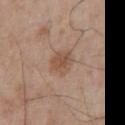notes: total-body-photography surveillance lesion; no biopsy
patient: male, roughly 65 years of age
site: the chest
lesion diameter: ≈3.5 mm
lighting: white-light
acquisition: ~15 mm tile from a whole-body skin photo
image-analysis metrics: a lesion area of about 7 mm², an eccentricity of roughly 0.65, and a shape-asymmetry score of about 0.2 (0 = symmetric); an average lesion color of about L≈52 a*≈18 b*≈28 (CIELAB) and a lesion-to-skin contrast of about 6.5 (normalized; higher = more distinct); a classifier nevus-likeness of about 50/100 and a lesion-detection confidence of about 100/100